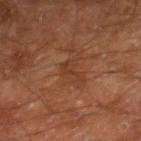  biopsy_status: not biopsied; imaged during a skin examination
  lighting: cross-polarized
  patient:
    sex: male
    age_approx: 60
  site: right leg
  image:
    source: total-body photography crop
    field_of_view_mm: 15
  automated_metrics:
    area_mm2_approx: 2.5
    eccentricity: 0.9
    shape_asymmetry: 0.6
    vs_skin_darker_L: 6.0
    vs_skin_contrast_norm: 5.5